From the left forearm; a lesion tile, about 15 mm wide, cut from a 3D total-body photograph; a female subject, in their 60s:
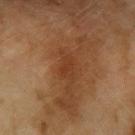Background: The lesion's longest dimension is about 2.5 mm. Captured under cross-polarized illumination. Diagnosis: Histopathologically confirmed as a melanoma in situ — a malignant skin lesion.